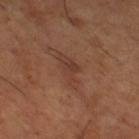Captured during whole-body skin photography for melanoma surveillance; the lesion was not biopsied. A close-up tile cropped from a whole-body skin photograph, about 15 mm across. From the right thigh. About 4.5 mm across. The tile uses cross-polarized illumination. A male patient approximately 65 years of age.